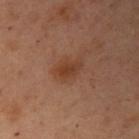Imaged during a routine full-body skin examination; the lesion was not biopsied and no histopathology is available. The lesion is located on the arm. The subject is a female approximately 55 years of age. This is a cross-polarized tile. Automated image analysis of the tile measured a footprint of about 3.5 mm², an outline eccentricity of about 0.85 (0 = round, 1 = elongated), and a shape-asymmetry score of about 0.35 (0 = symmetric). The analysis additionally found border irregularity of about 3 on a 0–10 scale and a color-variation rating of about 1/10. And it measured lesion-presence confidence of about 100/100. A 15 mm close-up extracted from a 3D total-body photography capture. The lesion's longest dimension is about 3 mm.